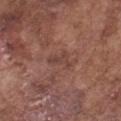Captured during whole-body skin photography for melanoma surveillance; the lesion was not biopsied. The tile uses white-light illumination. Longest diameter approximately 3 mm. A region of skin cropped from a whole-body photographic capture, roughly 15 mm wide. On the front of the torso. A male patient, in their mid- to late 70s.